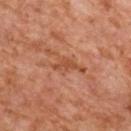The patient is a female roughly 60 years of age.
The lesion is on the upper back.
A region of skin cropped from a whole-body photographic capture, roughly 15 mm wide.
This is a cross-polarized tile.
The total-body-photography lesion software estimated a lesion color around L≈43 a*≈23 b*≈31 in CIELAB, a lesion–skin lightness drop of about 7, and a normalized border contrast of about 6. And it measured a border-irregularity index near 5/10, a color-variation rating of about 0/10, and radial color variation of about 0.